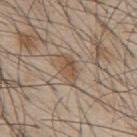Captured during whole-body skin photography for melanoma surveillance; the lesion was not biopsied.